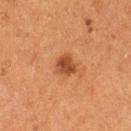Impression: Imaged during a routine full-body skin examination; the lesion was not biopsied and no histopathology is available. Acquisition and patient details: A female patient, aged approximately 50. On the right thigh. A region of skin cropped from a whole-body photographic capture, roughly 15 mm wide.